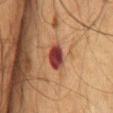Acquisition and patient details:
Measured at roughly 3 mm in maximum diameter. On the front of the torso. An algorithmic analysis of the crop reported a mean CIELAB color near L≈35 a*≈25 b*≈24 and a lesion–skin lightness drop of about 14. It also reported an automated nevus-likeness rating near 0 out of 100 and a lesion-detection confidence of about 100/100. Captured under cross-polarized illumination. The patient is a male about 65 years old. Cropped from a total-body skin-imaging series; the visible field is about 15 mm.